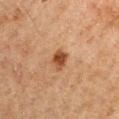workup — catalogued during a skin exam; not biopsied | image — 15 mm crop, total-body photography | body site — the arm | size — ≈2.5 mm | automated lesion analysis — a mean CIELAB color near L≈37 a*≈20 b*≈30, roughly 11 lightness units darker than nearby skin, and a normalized lesion–skin contrast near 9.5; a border-irregularity index near 2.5/10 and internal color variation of about 3 on a 0–10 scale; a nevus-likeness score of about 95/100 and lesion-presence confidence of about 100/100 | illumination — cross-polarized illumination | patient — male, aged 48 to 52.